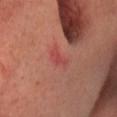| field | value |
|---|---|
| workup | total-body-photography surveillance lesion; no biopsy |
| subject | female, aged 58–62 |
| image source | ~15 mm crop, total-body skin-cancer survey |
| location | the head or neck |